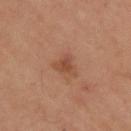Imaged during a routine full-body skin examination; the lesion was not biopsied and no histopathology is available. This image is a 15 mm lesion crop taken from a total-body photograph. About 3 mm across. The tile uses cross-polarized illumination. The patient is a male about 55 years old. Automated image analysis of the tile measured a lesion color around L≈47 a*≈22 b*≈31 in CIELAB, a lesion–skin lightness drop of about 8, and a lesion-to-skin contrast of about 6.5 (normalized; higher = more distinct). The analysis additionally found an automated nevus-likeness rating near 25 out of 100. The lesion is located on the back.